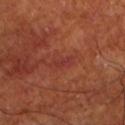follow-up: total-body-photography surveillance lesion; no biopsy | acquisition: ~15 mm crop, total-body skin-cancer survey | lesion diameter: ≈2.5 mm | illumination: cross-polarized | location: the left lower leg | TBP lesion metrics: a footprint of about 2.5 mm², an eccentricity of roughly 0.85, and a symmetry-axis asymmetry near 0.25; an average lesion color of about L≈35 a*≈29 b*≈27 (CIELAB); a border-irregularity index near 3/10, a within-lesion color-variation index near 0/10, and peripheral color asymmetry of about 0 | subject: male, aged around 70.